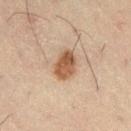<case>
<biopsy_status>not biopsied; imaged during a skin examination</biopsy_status>
<patient>
  <sex>male</sex>
  <age_approx>50</age_approx>
</patient>
<automated_metrics>
  <color_variation_0_10>4.0</color_variation_0_10>
  <peripheral_color_asymmetry>1.5</peripheral_color_asymmetry>
</automated_metrics>
<site>right thigh</site>
<lesion_size>
  <long_diameter_mm_approx>3.5</long_diameter_mm_approx>
</lesion_size>
<image>
  <source>total-body photography crop</source>
  <field_of_view_mm>15</field_of_view_mm>
</image>
<lighting>cross-polarized</lighting>
</case>